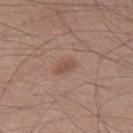Captured during whole-body skin photography for melanoma surveillance; the lesion was not biopsied.